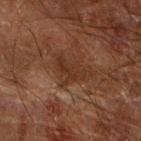notes — total-body-photography surveillance lesion; no biopsy
diameter — ~4.5 mm (longest diameter)
body site — the right forearm
illumination — cross-polarized illumination
imaging modality — ~15 mm crop, total-body skin-cancer survey
patient — male, aged 63–67
image-analysis metrics — a footprint of about 8 mm², an eccentricity of roughly 0.8, and a symmetry-axis asymmetry near 0.5; a border-irregularity index near 6.5/10 and radial color variation of about 1; a nevus-likeness score of about 0/100